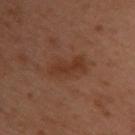Imaged during a routine full-body skin examination; the lesion was not biopsied and no histopathology is available. The lesion is on the back. Longest diameter approximately 5 mm. A region of skin cropped from a whole-body photographic capture, roughly 15 mm wide. The tile uses cross-polarized illumination. A female patient aged around 55.